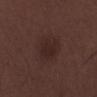{
  "biopsy_status": "not biopsied; imaged during a skin examination",
  "image": {
    "source": "total-body photography crop",
    "field_of_view_mm": 15
  },
  "patient": {
    "sex": "male",
    "age_approx": 70
  },
  "site": "left lower leg"
}